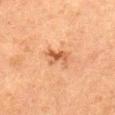{
  "biopsy_status": "not biopsied; imaged during a skin examination",
  "site": "abdomen",
  "patient": {
    "sex": "female",
    "age_approx": 50
  },
  "image": {
    "source": "total-body photography crop",
    "field_of_view_mm": 15
  },
  "lesion_size": {
    "long_diameter_mm_approx": 3.0
  }
}